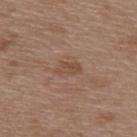{"biopsy_status": "not biopsied; imaged during a skin examination"}